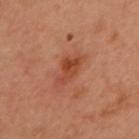biopsy status: imaged on a skin check; not biopsied
imaging modality: ~15 mm crop, total-body skin-cancer survey
subject: female, aged 58–62
site: the head or neck
tile lighting: cross-polarized illumination